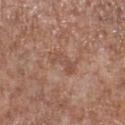biopsy_status: not biopsied; imaged during a skin examination
patient:
  sex: male
  age_approx: 55
image:
  source: total-body photography crop
  field_of_view_mm: 15
site: leg